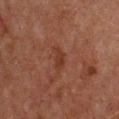{
  "biopsy_status": "not biopsied; imaged during a skin examination",
  "image": {
    "source": "total-body photography crop",
    "field_of_view_mm": 15
  },
  "lesion_size": {
    "long_diameter_mm_approx": 2.5
  },
  "automated_metrics": {
    "nevus_likeness_0_100": 5,
    "lesion_detection_confidence_0_100": 100
  },
  "site": "chest",
  "patient": {
    "sex": "male",
    "age_approx": 60
  }
}